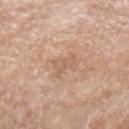notes: imaged on a skin check; not biopsied
tile lighting: white-light
site: the arm
patient: male, aged approximately 80
imaging modality: ~15 mm tile from a whole-body skin photo